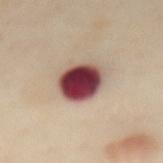Background: The lesion is located on the abdomen. The patient is a female in their mid-50s. This is a cross-polarized tile. A close-up tile cropped from a whole-body skin photograph, about 15 mm across. Measured at roughly 4 mm in maximum diameter.